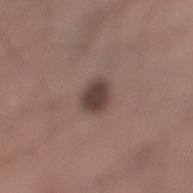Imaged during a routine full-body skin examination; the lesion was not biopsied and no histopathology is available. The patient is a male roughly 30 years of age. Automated image analysis of the tile measured a footprint of about 6 mm², an eccentricity of roughly 0.4, and a symmetry-axis asymmetry near 0.15. And it measured a border-irregularity index near 1.5/10, internal color variation of about 3.5 on a 0–10 scale, and radial color variation of about 1. The lesion is located on the right lower leg. A 15 mm close-up extracted from a 3D total-body photography capture. Imaged with white-light lighting. Approximately 2.5 mm at its widest.